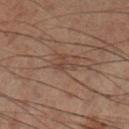workup: imaged on a skin check; not biopsied | automated metrics: an area of roughly 3 mm² and a shape eccentricity near 0.75; roughly 5 lightness units darker than nearby skin; a border-irregularity index near 5.5/10 | image source: ~15 mm tile from a whole-body skin photo | patient: male, roughly 50 years of age | anatomic site: the right lower leg | tile lighting: cross-polarized.